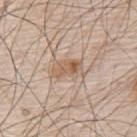A male subject roughly 80 years of age. A 15 mm crop from a total-body photograph taken for skin-cancer surveillance. About 3.5 mm across. This is a white-light tile. Located on the back.